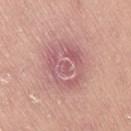Captured during whole-body skin photography for melanoma surveillance; the lesion was not biopsied. A female patient, about 45 years old. A roughly 15 mm field-of-view crop from a total-body skin photograph. From the right thigh. Captured under white-light illumination.